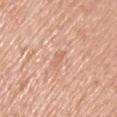Q: Was a biopsy performed?
A: total-body-photography surveillance lesion; no biopsy
Q: What is the anatomic site?
A: the front of the torso
Q: What are the patient's age and sex?
A: male, aged around 60
Q: Automated lesion metrics?
A: an area of roughly 2.5 mm², an outline eccentricity of about 0.9 (0 = round, 1 = elongated), and a symmetry-axis asymmetry near 0.3; a border-irregularity rating of about 3.5/10, internal color variation of about 0 on a 0–10 scale, and peripheral color asymmetry of about 0
Q: What kind of image is this?
A: total-body-photography crop, ~15 mm field of view
Q: How large is the lesion?
A: ≈3 mm